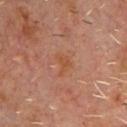biopsy status: imaged on a skin check; not biopsied
lesion diameter: about 3 mm
subject: male, approximately 60 years of age
acquisition: 15 mm crop, total-body photography
anatomic site: the chest
illumination: cross-polarized illumination
automated metrics: an average lesion color of about L≈47 a*≈23 b*≈33 (CIELAB) and a normalized border contrast of about 6; a classifier nevus-likeness of about 0/100 and a detector confidence of about 100 out of 100 that the crop contains a lesion